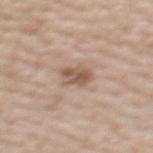imaging modality — ~15 mm tile from a whole-body skin photo
diameter — about 3.5 mm
location — the upper back
subject — female, aged around 60